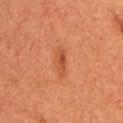Case summary:
– notes — total-body-photography surveillance lesion; no biopsy
– anatomic site — the upper back
– illumination — cross-polarized
– image — ~15 mm tile from a whole-body skin photo
– subject — female, about 65 years old
– image-analysis metrics — a footprint of about 3 mm², an outline eccentricity of about 0.95 (0 = round, 1 = elongated), and a symmetry-axis asymmetry near 0.25; an average lesion color of about L≈50 a*≈30 b*≈39 (CIELAB), a lesion–skin lightness drop of about 9, and a normalized lesion–skin contrast near 6.5; a nevus-likeness score of about 15/100
– size — ~3 mm (longest diameter)